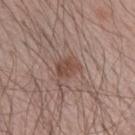{"biopsy_status": "not biopsied; imaged during a skin examination", "image": {"source": "total-body photography crop", "field_of_view_mm": 15}, "patient": {"sex": "male", "age_approx": 30}, "site": "left forearm"}